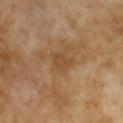Acquisition and patient details: A female subject aged 58 to 62. The lesion is on the back. A close-up tile cropped from a whole-body skin photograph, about 15 mm across. Measured at roughly 3 mm in maximum diameter. An algorithmic analysis of the crop reported a border-irregularity rating of about 3/10, a color-variation rating of about 1.5/10, and radial color variation of about 0.5. The software also gave a nevus-likeness score of about 0/100 and a detector confidence of about 100 out of 100 that the crop contains a lesion.